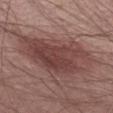lesion diameter = ≈10 mm
patient = male, in their mid- to late 60s
lighting = white-light illumination
site = the abdomen
image source = ~15 mm crop, total-body skin-cancer survey
TBP lesion metrics = an average lesion color of about L≈43 a*≈21 b*≈21 (CIELAB); a nevus-likeness score of about 70/100 and lesion-presence confidence of about 100/100